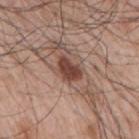Clinical impression: The lesion was tiled from a total-body skin photograph and was not biopsied. Acquisition and patient details: The recorded lesion diameter is about 3.5 mm. This is a white-light tile. On the right upper arm. A male patient, aged 63 to 67. A 15 mm close-up extracted from a 3D total-body photography capture.